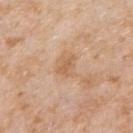Q: Was a biopsy performed?
A: catalogued during a skin exam; not biopsied
Q: Where on the body is the lesion?
A: the right upper arm
Q: Lesion size?
A: about 2.5 mm
Q: What are the patient's age and sex?
A: male, aged approximately 75
Q: How was the tile lit?
A: white-light illumination
Q: How was this image acquired?
A: ~15 mm tile from a whole-body skin photo